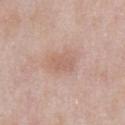Assessment:
Part of a total-body skin-imaging series; this lesion was reviewed on a skin check and was not flagged for biopsy.
Clinical summary:
The total-body-photography lesion software estimated a footprint of about 12 mm², an outline eccentricity of about 0.75 (0 = round, 1 = elongated), and a symmetry-axis asymmetry near 0.25. The software also gave an automated nevus-likeness rating near 0 out of 100. The subject is a male aged 53 to 57. Located on the abdomen. The tile uses white-light illumination. Approximately 5 mm at its widest. This image is a 15 mm lesion crop taken from a total-body photograph.